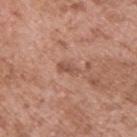Case summary:
– follow-up · catalogued during a skin exam; not biopsied
– location · the back
– diameter · ≈2.5 mm
– illumination · white-light illumination
– automated lesion analysis · an area of roughly 3 mm², an eccentricity of roughly 0.85, and a shape-asymmetry score of about 0.3 (0 = symmetric); an average lesion color of about L≈52 a*≈22 b*≈28 (CIELAB) and a normalized border contrast of about 6
– subject · male, aged around 55
– acquisition · total-body-photography crop, ~15 mm field of view